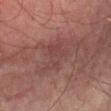workup = total-body-photography surveillance lesion; no biopsy | patient = male, aged 73 to 77 | imaging modality = 15 mm crop, total-body photography | lighting = cross-polarized illumination | location = the left lower leg | automated metrics = a border-irregularity index near 5.5/10; a classifier nevus-likeness of about 0/100 | lesion size = about 5 mm.